A female patient, aged 68 to 72 · this image is a 15 mm lesion crop taken from a total-body photograph · this is a white-light tile · the lesion is located on the left forearm · the lesion's longest dimension is about 4 mm — 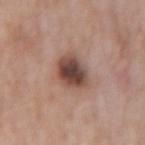Diagnosis:
Biopsy histopathology demonstrated a benign skin lesion: dysplastic (Clark) nevus.